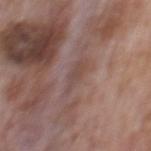Case summary:
* follow-up: total-body-photography surveillance lesion; no biopsy
* lighting: white-light
* patient: male, roughly 70 years of age
* lesion size: ≈3.5 mm
* body site: the mid back
* automated lesion analysis: roughly 6 lightness units darker than nearby skin; an automated nevus-likeness rating near 0 out of 100
* acquisition: total-body-photography crop, ~15 mm field of view